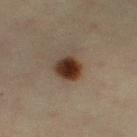biopsy status: no biopsy performed (imaged during a skin exam); lesion diameter: ≈3 mm; imaging modality: ~15 mm crop, total-body skin-cancer survey; lighting: cross-polarized illumination; body site: the right thigh; subject: female, aged approximately 45.